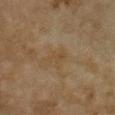Captured during whole-body skin photography for melanoma surveillance; the lesion was not biopsied.
The subject is a female aged 58–62.
A 15 mm crop from a total-body photograph taken for skin-cancer surveillance.
Measured at roughly 3 mm in maximum diameter.
The tile uses cross-polarized illumination.
On the chest.